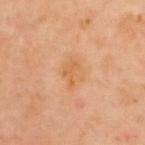<tbp_lesion>
<biopsy_status>not biopsied; imaged during a skin examination</biopsy_status>
<lesion_size>
  <long_diameter_mm_approx>3.5</long_diameter_mm_approx>
</lesion_size>
<lighting>cross-polarized</lighting>
<image>
  <source>total-body photography crop</source>
  <field_of_view_mm>15</field_of_view_mm>
</image>
<site>back</site>
<patient>
  <sex>male</sex>
  <age_approx>65</age_approx>
</patient>
</tbp_lesion>